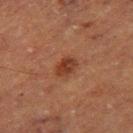| key | value |
|---|---|
| follow-up | total-body-photography surveillance lesion; no biopsy |
| image source | 15 mm crop, total-body photography |
| body site | the right thigh |
| patient | male, in their mid- to late 70s |
| automated metrics | an area of roughly 4.5 mm² and a shape-asymmetry score of about 0.2 (0 = symmetric); radial color variation of about 1 |
| illumination | cross-polarized illumination |